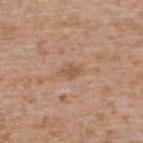Captured during whole-body skin photography for melanoma surveillance; the lesion was not biopsied.
Captured under white-light illumination.
A 15 mm close-up tile from a total-body photography series done for melanoma screening.
From the upper back.
The recorded lesion diameter is about 3 mm.
The patient is a male in their mid- to late 60s.